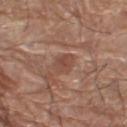Q: Is there a histopathology result?
A: no biopsy performed (imaged during a skin exam)
Q: Patient demographics?
A: male, roughly 70 years of age
Q: What is the anatomic site?
A: the leg
Q: Illumination type?
A: white-light
Q: What is the lesion's diameter?
A: about 2.5 mm
Q: What kind of image is this?
A: 15 mm crop, total-body photography
Q: What did automated image analysis measure?
A: an area of roughly 4.5 mm²; a mean CIELAB color near L≈47 a*≈21 b*≈27 and a lesion-to-skin contrast of about 6 (normalized; higher = more distinct); a classifier nevus-likeness of about 20/100 and lesion-presence confidence of about 95/100